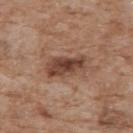Recorded during total-body skin imaging; not selected for excision or biopsy.
Captured under white-light illumination.
The recorded lesion diameter is about 5 mm.
A 15 mm close-up extracted from a 3D total-body photography capture.
Located on the chest.
The subject is a male roughly 60 years of age.